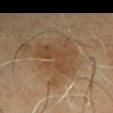Captured during whole-body skin photography for melanoma surveillance; the lesion was not biopsied. The lesion-visualizer software estimated a mean CIELAB color near L≈35 a*≈14 b*≈27, roughly 5 lightness units darker than nearby skin, and a lesion-to-skin contrast of about 5.5 (normalized; higher = more distinct). It also reported a border-irregularity index near 7/10, a within-lesion color-variation index near 2/10, and radial color variation of about 1. It also reported a classifier nevus-likeness of about 5/100. On the arm. A male patient about 70 years old. Imaged with cross-polarized lighting. This image is a 15 mm lesion crop taken from a total-body photograph.